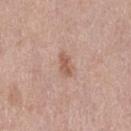Imaged during a routine full-body skin examination; the lesion was not biopsied and no histopathology is available. The patient is a female about 40 years old. Cropped from a whole-body photographic skin survey; the tile spans about 15 mm. The lesion is located on the left thigh.